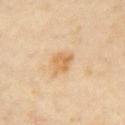The lesion was photographed on a routine skin check and not biopsied; there is no pathology result.
An algorithmic analysis of the crop reported a lesion area of about 5 mm², an eccentricity of roughly 0.65, and a shape-asymmetry score of about 0.25 (0 = symmetric). It also reported a border-irregularity rating of about 2.5/10 and peripheral color asymmetry of about 1.5.
A female subject, in their mid-30s.
Captured under cross-polarized illumination.
The lesion is located on the chest.
Cropped from a total-body skin-imaging series; the visible field is about 15 mm.